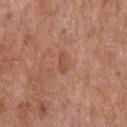Imaged during a routine full-body skin examination; the lesion was not biopsied and no histopathology is available.
The tile uses white-light illumination.
From the chest.
A male subject aged around 65.
Automated image analysis of the tile measured an area of roughly 2.5 mm² and an outline eccentricity of about 0.85 (0 = round, 1 = elongated). And it measured an average lesion color of about L≈50 a*≈24 b*≈31 (CIELAB), roughly 7 lightness units darker than nearby skin, and a normalized lesion–skin contrast near 5.5. It also reported a nevus-likeness score of about 0/100 and a detector confidence of about 100 out of 100 that the crop contains a lesion.
A lesion tile, about 15 mm wide, cut from a 3D total-body photograph.